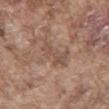Part of a total-body skin-imaging series; this lesion was reviewed on a skin check and was not flagged for biopsy. A male subject, roughly 75 years of age. An algorithmic analysis of the crop reported a lesion area of about 5.5 mm², a shape eccentricity near 0.9, and two-axis asymmetry of about 0.4. It also reported an average lesion color of about L≈50 a*≈17 b*≈26 (CIELAB) and about 7 CIELAB-L* units darker than the surrounding skin. The software also gave a border-irregularity rating of about 6/10 and a within-lesion color-variation index near 2.5/10. A region of skin cropped from a whole-body photographic capture, roughly 15 mm wide. Captured under white-light illumination. The lesion is located on the back. Longest diameter approximately 4 mm.